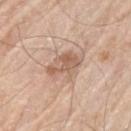Imaged during a routine full-body skin examination; the lesion was not biopsied and no histopathology is available. The lesion is located on the left upper arm. The recorded lesion diameter is about 4 mm. This is a white-light tile. A 15 mm close-up tile from a total-body photography series done for melanoma screening. A male patient roughly 65 years of age.